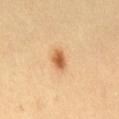workup=total-body-photography surveillance lesion; no biopsy | subject=female, aged approximately 30 | lesion size=~2.5 mm (longest diameter) | body site=the mid back | image source=total-body-photography crop, ~15 mm field of view | lighting=cross-polarized illumination.